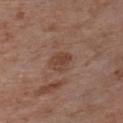Case summary:
* follow-up — total-body-photography surveillance lesion; no biopsy
* patient — male, roughly 60 years of age
* image — ~15 mm crop, total-body skin-cancer survey
* anatomic site — the chest
* lighting — white-light illumination